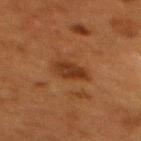<lesion>
<biopsy_status>not biopsied; imaged during a skin examination</biopsy_status>
<lighting>cross-polarized</lighting>
<site>mid back</site>
<automated_metrics>
  <vs_skin_darker_L>8.0</vs_skin_darker_L>
  <vs_skin_contrast_norm>8.0</vs_skin_contrast_norm>
  <color_variation_0_10>3.0</color_variation_0_10>
  <peripheral_color_asymmetry>1.0</peripheral_color_asymmetry>
  <nevus_likeness_0_100>65</nevus_likeness_0_100>
</automated_metrics>
<image>
  <source>total-body photography crop</source>
  <field_of_view_mm>15</field_of_view_mm>
</image>
<lesion_size>
  <long_diameter_mm_approx>4.0</long_diameter_mm_approx>
</lesion_size>
<patient>
  <sex>female</sex>
  <age_approx>50</age_approx>
</patient>
</lesion>